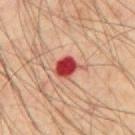workup = no biopsy performed (imaged during a skin exam) | patient = male, aged around 45 | size = about 3.5 mm | illumination = cross-polarized | site = the chest | acquisition = 15 mm crop, total-body photography.